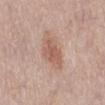Clinical impression:
Recorded during total-body skin imaging; not selected for excision or biopsy.
Image and clinical context:
A lesion tile, about 15 mm wide, cut from a 3D total-body photograph. The tile uses white-light illumination. The recorded lesion diameter is about 4 mm. The lesion is located on the left lower leg. A female subject, roughly 65 years of age.